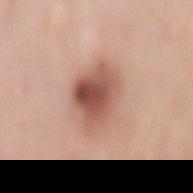workup=no biopsy performed (imaged during a skin exam)